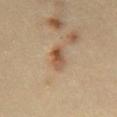<tbp_lesion>
  <biopsy_status>not biopsied; imaged during a skin examination</biopsy_status>
  <patient>
    <sex>male</sex>
    <age_approx>35</age_approx>
  </patient>
  <image>
    <source>total-body photography crop</source>
    <field_of_view_mm>15</field_of_view_mm>
  </image>
  <site>front of the torso</site>
</tbp_lesion>